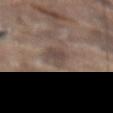Recorded during total-body skin imaging; not selected for excision or biopsy. Cropped from a whole-body photographic skin survey; the tile spans about 15 mm. The total-body-photography lesion software estimated a footprint of about 6.5 mm², an eccentricity of roughly 0.8, and a shape-asymmetry score of about 0.25 (0 = symmetric). About 4 mm across. The lesion is on the abdomen. A male patient, aged around 80. The tile uses white-light illumination.